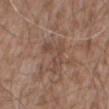| key | value |
|---|---|
| notes | total-body-photography surveillance lesion; no biopsy |
| body site | the mid back |
| illumination | white-light illumination |
| patient | male, in their mid-50s |
| image source | ~15 mm crop, total-body skin-cancer survey |
| lesion size | ≈4.5 mm |